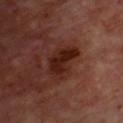  biopsy_status: not biopsied; imaged during a skin examination
  image:
    source: total-body photography crop
    field_of_view_mm: 15
  lighting: cross-polarized
  automated_metrics:
    cielab_L: 23
    cielab_a: 22
    cielab_b: 24
    vs_skin_darker_L: 9.0
    vs_skin_contrast_norm: 10.0
  site: chest
  patient:
    sex: male
    age_approx: 70